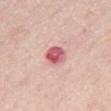The lesion was tiled from a total-body skin photograph and was not biopsied.
This is a white-light tile.
Cropped from a whole-body photographic skin survey; the tile spans about 15 mm.
About 3 mm across.
A male subject, about 60 years old.
The lesion is on the abdomen.